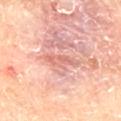Part of a total-body skin-imaging series; this lesion was reviewed on a skin check and was not flagged for biopsy.